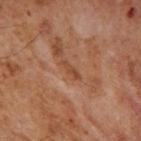Q: Was this lesion biopsied?
A: total-body-photography surveillance lesion; no biopsy
Q: Where on the body is the lesion?
A: the right upper arm
Q: How was this image acquired?
A: ~15 mm crop, total-body skin-cancer survey
Q: Lesion size?
A: about 3 mm
Q: Who is the patient?
A: male, in their mid- to late 50s
Q: What lighting was used for the tile?
A: cross-polarized illumination